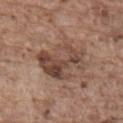| field | value |
|---|---|
| follow-up | catalogued during a skin exam; not biopsied |
| patient | male, approximately 80 years of age |
| acquisition | ~15 mm tile from a whole-body skin photo |
| lighting | white-light |
| size | ≈6.5 mm |
| body site | the back |